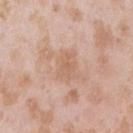| feature | finding |
|---|---|
| workup | catalogued during a skin exam; not biopsied |
| acquisition | 15 mm crop, total-body photography |
| lesion diameter | ~3 mm (longest diameter) |
| subject | female, approximately 25 years of age |
| body site | the right upper arm |
| TBP lesion metrics | a shape eccentricity near 0.85 and a shape-asymmetry score of about 0.35 (0 = symmetric); an average lesion color of about L≈62 a*≈20 b*≈31 (CIELAB), a lesion–skin lightness drop of about 6, and a normalized border contrast of about 5; an automated nevus-likeness rating near 0 out of 100 |
| lighting | white-light |